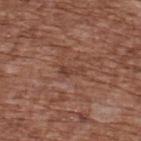Image and clinical context: Measured at roughly 3 mm in maximum diameter. A 15 mm close-up tile from a total-body photography series done for melanoma screening. The lesion is located on the upper back. A male subject in their mid- to late 70s. An algorithmic analysis of the crop reported an area of roughly 2.5 mm², an eccentricity of roughly 0.9, and a symmetry-axis asymmetry near 0.4. And it measured a border-irregularity index near 4.5/10. The tile uses white-light illumination.